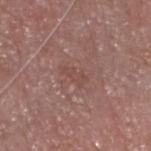{
  "biopsy_status": "not biopsied; imaged during a skin examination",
  "lesion_size": {
    "long_diameter_mm_approx": 3.0
  },
  "image": {
    "source": "total-body photography crop",
    "field_of_view_mm": 15
  },
  "lighting": "white-light",
  "site": "head or neck",
  "patient": {
    "sex": "male",
    "age_approx": 75
  },
  "automated_metrics": {
    "area_mm2_approx": 3.0,
    "eccentricity": 0.9,
    "shape_asymmetry": 0.35,
    "cielab_L": 47,
    "cielab_a": 20,
    "cielab_b": 23,
    "vs_skin_contrast_norm": 4.5,
    "border_irregularity_0_10": 4.0,
    "color_variation_0_10": 0.0,
    "peripheral_color_asymmetry": 0.0,
    "nevus_likeness_0_100": 0,
    "lesion_detection_confidence_0_100": 100
  }
}